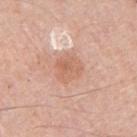This image is a 15 mm lesion crop taken from a total-body photograph.
About 3.5 mm across.
From the arm.
The patient is a male roughly 80 years of age.